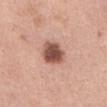  biopsy_status: not biopsied; imaged during a skin examination
  site: abdomen
  lesion_size:
    long_diameter_mm_approx: 3.5
  automated_metrics:
    area_mm2_approx: 9.0
    eccentricity: 0.4
    shape_asymmetry: 0.2
    border_irregularity_0_10: 2.0
    color_variation_0_10: 4.5
    peripheral_color_asymmetry: 1.0
    nevus_likeness_0_100: 80
    lesion_detection_confidence_0_100: 100
  patient:
    sex: female
    age_approx: 40
  image:
    source: total-body photography crop
    field_of_view_mm: 15
  lighting: white-light